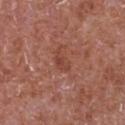Assessment: This lesion was catalogued during total-body skin photography and was not selected for biopsy. Context: A male patient in their mid-60s. Located on the chest. An algorithmic analysis of the crop reported an area of roughly 3.5 mm² and a shape-asymmetry score of about 0.3 (0 = symmetric). It also reported a mean CIELAB color near L≈44 a*≈26 b*≈29, about 7 CIELAB-L* units darker than the surrounding skin, and a normalized lesion–skin contrast near 5.5. The analysis additionally found a border-irregularity rating of about 3/10 and a peripheral color-asymmetry measure near 0.5. The tile uses white-light illumination. The recorded lesion diameter is about 2.5 mm. Cropped from a total-body skin-imaging series; the visible field is about 15 mm.